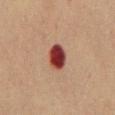| feature | finding |
|---|---|
| follow-up | catalogued during a skin exam; not biopsied |
| lighting | cross-polarized illumination |
| image source | total-body-photography crop, ~15 mm field of view |
| site | the mid back |
| subject | female, aged around 70 |